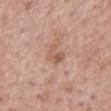This lesion was catalogued during total-body skin photography and was not selected for biopsy. A 15 mm crop from a total-body photograph taken for skin-cancer surveillance. The lesion is on the mid back. Captured under white-light illumination. A male subject, aged approximately 65. Approximately 3 mm at its widest.